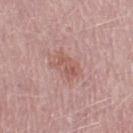  biopsy_status: not biopsied; imaged during a skin examination
  site: leg
  patient:
    sex: male
    age_approx: 50
  image:
    source: total-body photography crop
    field_of_view_mm: 15
  lesion_size:
    long_diameter_mm_approx: 3.5
  lighting: white-light